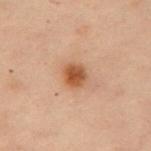Case summary:
– notes · imaged on a skin check; not biopsied
– site · the chest
– subject · female, about 55 years old
– image · ~15 mm tile from a whole-body skin photo
– illumination · cross-polarized
– lesion size · ≈3 mm